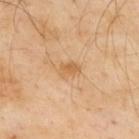biopsy status: imaged on a skin check; not biopsied | patient: male, in their mid-50s | automated lesion analysis: a mean CIELAB color near L≈62 a*≈20 b*≈41 and a normalized lesion–skin contrast near 6.5; a classifier nevus-likeness of about 10/100 and lesion-presence confidence of about 100/100 | illumination: cross-polarized illumination | location: the upper back | size: ~2.5 mm (longest diameter) | imaging modality: ~15 mm crop, total-body skin-cancer survey.